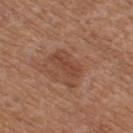<lesion>
  <biopsy_status>not biopsied; imaged during a skin examination</biopsy_status>
  <patient>
    <sex>female</sex>
    <age_approx>65</age_approx>
  </patient>
  <lesion_size>
    <long_diameter_mm_approx>4.5</long_diameter_mm_approx>
  </lesion_size>
  <site>left leg</site>
  <lighting>white-light</lighting>
  <image>
    <source>total-body photography crop</source>
    <field_of_view_mm>15</field_of_view_mm>
  </image>
</lesion>